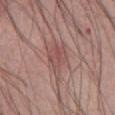workup = no biopsy performed (imaged during a skin exam); diameter = about 5 mm; site = the abdomen; illumination = white-light; patient = male, in their mid- to late 50s; image = total-body-photography crop, ~15 mm field of view.